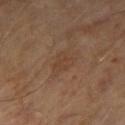{
  "biopsy_status": "not biopsied; imaged during a skin examination",
  "automated_metrics": {
    "area_mm2_approx": 5.0,
    "eccentricity": 0.7,
    "cielab_L": 39,
    "cielab_a": 17,
    "cielab_b": 28,
    "vs_skin_darker_L": 5.0,
    "vs_skin_contrast_norm": 4.5,
    "nevus_likeness_0_100": 0
  },
  "image": {
    "source": "total-body photography crop",
    "field_of_view_mm": 15
  },
  "patient": {
    "sex": "male",
    "age_approx": 65
  }
}